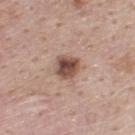A region of skin cropped from a whole-body photographic capture, roughly 15 mm wide.
The subject is a male aged approximately 45.
This is a white-light tile.
Located on the back.
The total-body-photography lesion software estimated a footprint of about 6.5 mm², an outline eccentricity of about 0.4 (0 = round, 1 = elongated), and a symmetry-axis asymmetry near 0.2. And it measured a normalized border contrast of about 10.5. The analysis additionally found a lesion-detection confidence of about 100/100.
About 3 mm across.